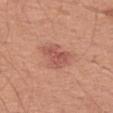Findings:
* body site — the left thigh
* imaging modality — ~15 mm tile from a whole-body skin photo
* patient — male, about 65 years old